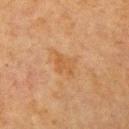follow-up = catalogued during a skin exam; not biopsied
anatomic site = the left upper arm
lesion size = ~4 mm (longest diameter)
acquisition = total-body-photography crop, ~15 mm field of view
patient = female, aged approximately 55
lighting = cross-polarized illumination